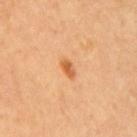Assessment:
Part of a total-body skin-imaging series; this lesion was reviewed on a skin check and was not flagged for biopsy.
Background:
The lesion is located on the upper back. A close-up tile cropped from a whole-body skin photograph, about 15 mm across. Captured under cross-polarized illumination. A female subject, aged around 65.